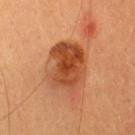Assessment:
Imaged during a routine full-body skin examination; the lesion was not biopsied and no histopathology is available.
Image and clinical context:
About 6.5 mm across. A female subject aged around 30. A region of skin cropped from a whole-body photographic capture, roughly 15 mm wide. Imaged with cross-polarized lighting. Automated tile analysis of the lesion measured a classifier nevus-likeness of about 65/100 and lesion-presence confidence of about 100/100. The lesion is located on the head or neck.